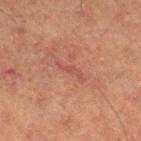Assessment: Imaged during a routine full-body skin examination; the lesion was not biopsied and no histopathology is available. Image and clinical context: Captured under cross-polarized illumination. Cropped from a whole-body photographic skin survey; the tile spans about 15 mm. Located on the leg. A female subject, aged around 60. About 3.5 mm across.